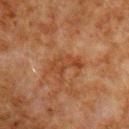Recorded during total-body skin imaging; not selected for excision or biopsy. A male subject, about 80 years old. A close-up tile cropped from a whole-body skin photograph, about 15 mm across. From the right upper arm. The recorded lesion diameter is about 4 mm. Imaged with cross-polarized lighting. The lesion-visualizer software estimated a lesion area of about 6.5 mm², an eccentricity of roughly 0.85, and a symmetry-axis asymmetry near 0.4. The analysis additionally found a lesion color around L≈34 a*≈21 b*≈31 in CIELAB and a normalized border contrast of about 6. It also reported a nevus-likeness score of about 0/100 and a detector confidence of about 100 out of 100 that the crop contains a lesion.